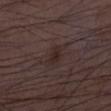Notes:
* workup: catalogued during a skin exam; not biopsied
* patient: male, in their 50s
* acquisition: total-body-photography crop, ~15 mm field of view
* site: the left lower leg
* size: ≈2.5 mm
* image-analysis metrics: a border-irregularity rating of about 2.5/10, a within-lesion color-variation index near 1/10, and peripheral color asymmetry of about 0.5